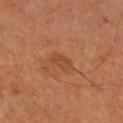Notes:
• follow-up — no biopsy performed (imaged during a skin exam)
• automated metrics — an outline eccentricity of about 0.8 (0 = round, 1 = elongated) and two-axis asymmetry of about 0.25; a lesion–skin lightness drop of about 7; a border-irregularity index near 2/10, a color-variation rating of about 2/10, and a peripheral color-asymmetry measure near 1
• patient — male, in their mid- to late 60s
• tile lighting — cross-polarized
• image source — ~15 mm crop, total-body skin-cancer survey
• body site — the right upper arm
• lesion diameter — about 2.5 mm